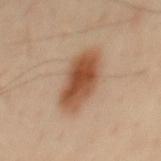Impression:
Part of a total-body skin-imaging series; this lesion was reviewed on a skin check and was not flagged for biopsy.
Context:
Measured at roughly 6.5 mm in maximum diameter. A male patient approximately 45 years of age. Captured under cross-polarized illumination. The lesion is located on the mid back. A roughly 15 mm field-of-view crop from a total-body skin photograph.